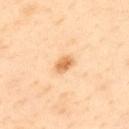Assessment: The lesion was tiled from a total-body skin photograph and was not biopsied. Acquisition and patient details: A 15 mm close-up tile from a total-body photography series done for melanoma screening. Longest diameter approximately 2.5 mm. Automated image analysis of the tile measured a lesion color around L≈71 a*≈23 b*≈44 in CIELAB and a lesion–skin lightness drop of about 13. The analysis additionally found border irregularity of about 2 on a 0–10 scale and internal color variation of about 2.5 on a 0–10 scale. The lesion is located on the back. The subject is a male aged 53–57.